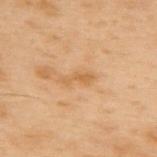Clinical impression: Captured during whole-body skin photography for melanoma surveillance; the lesion was not biopsied. Acquisition and patient details: The tile uses cross-polarized illumination. A 15 mm close-up extracted from a 3D total-body photography capture. A male patient, aged around 55. Approximately 3 mm at its widest. On the upper back. Automated image analysis of the tile measured a lesion color around L≈49 a*≈18 b*≈35 in CIELAB and roughly 6 lightness units darker than nearby skin. The software also gave an automated nevus-likeness rating near 0 out of 100.